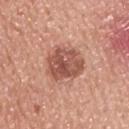Clinical impression: Captured during whole-body skin photography for melanoma surveillance; the lesion was not biopsied. Clinical summary: Approximately 4.5 mm at its widest. On the upper back. A 15 mm close-up tile from a total-body photography series done for melanoma screening. Captured under white-light illumination. The subject is a male about 65 years old. Automated tile analysis of the lesion measured an average lesion color of about L≈53 a*≈25 b*≈28 (CIELAB), a lesion–skin lightness drop of about 13, and a lesion-to-skin contrast of about 8.5 (normalized; higher = more distinct). The analysis additionally found a border-irregularity rating of about 2/10 and a within-lesion color-variation index near 5.5/10.